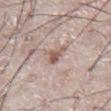{"biopsy_status": "not biopsied; imaged during a skin examination", "site": "abdomen", "patient": {"sex": "male", "age_approx": 75}, "lighting": "white-light", "automated_metrics": {"area_mm2_approx": 4.5, "eccentricity": 0.55, "shape_asymmetry": 0.5}, "lesion_size": {"long_diameter_mm_approx": 2.5}, "image": {"source": "total-body photography crop", "field_of_view_mm": 15}}